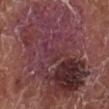Q: Is there a histopathology result?
A: no biopsy performed (imaged during a skin exam)
Q: Lesion location?
A: the leg
Q: What is the imaging modality?
A: ~15 mm crop, total-body skin-cancer survey
Q: What is the lesion's diameter?
A: ≈13 mm
Q: What did automated image analysis measure?
A: a lesion area of about 85 mm², an eccentricity of roughly 0.75, and a shape-asymmetry score of about 0.25 (0 = symmetric); a mean CIELAB color near L≈36 a*≈24 b*≈16, about 9 CIELAB-L* units darker than the surrounding skin, and a normalized border contrast of about 9; a classifier nevus-likeness of about 0/100
Q: What are the patient's age and sex?
A: male, in their mid- to late 70s